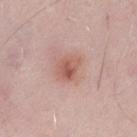{"site": "left thigh", "image": {"source": "total-body photography crop", "field_of_view_mm": 15}, "lighting": "white-light", "patient": {"sex": "male", "age_approx": 50}}